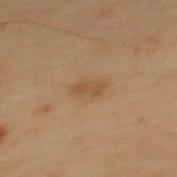No biopsy was performed on this lesion — it was imaged during a full skin examination and was not determined to be concerning. A male subject, about 55 years old. A lesion tile, about 15 mm wide, cut from a 3D total-body photograph. Located on the mid back.